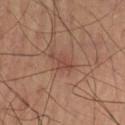The lesion was photographed on a routine skin check and not biopsied; there is no pathology result. Located on the left thigh. Automated tile analysis of the lesion measured an area of roughly 4 mm², an outline eccentricity of about 0.85 (0 = round, 1 = elongated), and a symmetry-axis asymmetry near 0.4. It also reported a mean CIELAB color near L≈43 a*≈21 b*≈26, roughly 7 lightness units darker than nearby skin, and a normalized border contrast of about 5.5. A male subject aged around 70. Imaged with cross-polarized lighting. Measured at roughly 3 mm in maximum diameter. This image is a 15 mm lesion crop taken from a total-body photograph.